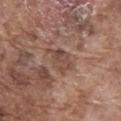Assessment:
This lesion was catalogued during total-body skin photography and was not selected for biopsy.
Image and clinical context:
A male patient aged approximately 75. Approximately 4 mm at its widest. The lesion is located on the abdomen. A close-up tile cropped from a whole-body skin photograph, about 15 mm across.